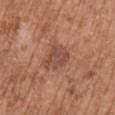No biopsy was performed on this lesion — it was imaged during a full skin examination and was not determined to be concerning.
A close-up tile cropped from a whole-body skin photograph, about 15 mm across.
A female patient, aged around 75.
The lesion is located on the right upper arm.